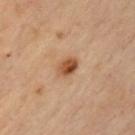diameter = about 3 mm; patient = male, about 55 years old; site = the chest; tile lighting = cross-polarized illumination; imaging modality = ~15 mm crop, total-body skin-cancer survey.